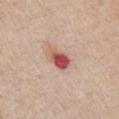{
  "biopsy_status": "not biopsied; imaged during a skin examination",
  "lesion_size": {
    "long_diameter_mm_approx": 3.0
  },
  "image": {
    "source": "total-body photography crop",
    "field_of_view_mm": 15
  },
  "patient": {
    "sex": "male",
    "age_approx": 60
  },
  "lighting": "white-light",
  "site": "right upper arm"
}